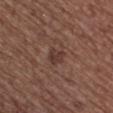{"biopsy_status": "not biopsied; imaged during a skin examination", "patient": {"sex": "female", "age_approx": 75}, "site": "upper back", "image": {"source": "total-body photography crop", "field_of_view_mm": 15}, "lighting": "white-light", "automated_metrics": {"area_mm2_approx": 3.5, "eccentricity": 0.75, "border_irregularity_0_10": 3.0, "color_variation_0_10": 1.5, "peripheral_color_asymmetry": 0.5, "nevus_likeness_0_100": 5, "lesion_detection_confidence_0_100": 100}, "lesion_size": {"long_diameter_mm_approx": 2.5}}